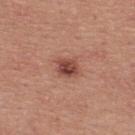The lesion was tiled from a total-body skin photograph and was not biopsied. From the upper back. A 15 mm crop from a total-body photograph taken for skin-cancer surveillance. The patient is a male aged around 40.